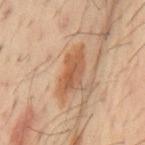Clinical impression: No biopsy was performed on this lesion — it was imaged during a full skin examination and was not determined to be concerning. Context: A male subject aged 58–62. A region of skin cropped from a whole-body photographic capture, roughly 15 mm wide. On the mid back. Automated tile analysis of the lesion measured an area of roughly 11 mm² and an eccentricity of roughly 0.9. The software also gave a border-irregularity rating of about 4/10, internal color variation of about 3.5 on a 0–10 scale, and peripheral color asymmetry of about 1. Imaged with cross-polarized lighting.